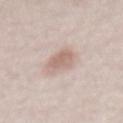Imaged during a routine full-body skin examination; the lesion was not biopsied and no histopathology is available.
A close-up tile cropped from a whole-body skin photograph, about 15 mm across.
On the abdomen.
The patient is a female aged around 50.